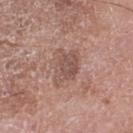notes=catalogued during a skin exam; not biopsied
image source=15 mm crop, total-body photography
location=the right thigh
subject=male, aged around 75
size=~4.5 mm (longest diameter)
illumination=white-light illumination